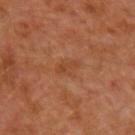{
  "biopsy_status": "not biopsied; imaged during a skin examination",
  "patient": {
    "sex": "male",
    "age_approx": 30
  },
  "site": "left forearm",
  "automated_metrics": {
    "cielab_L": 43,
    "cielab_a": 24,
    "cielab_b": 34,
    "vs_skin_darker_L": 6.0,
    "border_irregularity_0_10": 4.0,
    "color_variation_0_10": 0.0
  },
  "image": {
    "source": "total-body photography crop",
    "field_of_view_mm": 15
  },
  "lighting": "cross-polarized",
  "lesion_size": {
    "long_diameter_mm_approx": 3.0
  }
}